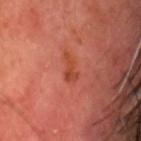| key | value |
|---|---|
| biopsy status | imaged on a skin check; not biopsied |
| anatomic site | the head or neck |
| illumination | cross-polarized illumination |
| subject | male, about 70 years old |
| lesion diameter | about 3 mm |
| acquisition | total-body-photography crop, ~15 mm field of view |